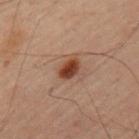Notes:
- notes: total-body-photography surveillance lesion; no biopsy
- image: ~15 mm tile from a whole-body skin photo
- tile lighting: cross-polarized
- location: the left upper arm
- subject: male, approximately 60 years of age